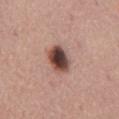Findings:
* lesion diameter · about 4 mm
* imaging modality · ~15 mm crop, total-body skin-cancer survey
* patient · male, aged 73–77
* location · the abdomen
* automated lesion analysis · an area of roughly 8.5 mm², a shape eccentricity near 0.7, and two-axis asymmetry of about 0.15; border irregularity of about 1.5 on a 0–10 scale, a color-variation rating of about 8.5/10, and peripheral color asymmetry of about 2.5; a classifier nevus-likeness of about 80/100 and a lesion-detection confidence of about 100/100